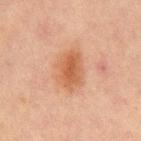Clinical impression:
Recorded during total-body skin imaging; not selected for excision or biopsy.
Clinical summary:
A close-up tile cropped from a whole-body skin photograph, about 15 mm across. Automated tile analysis of the lesion measured a lesion area of about 11 mm² and an eccentricity of roughly 0.7. The analysis additionally found a mean CIELAB color near L≈47 a*≈21 b*≈31, about 8 CIELAB-L* units darker than the surrounding skin, and a normalized border contrast of about 7.5. And it measured a nevus-likeness score of about 85/100 and a lesion-detection confidence of about 100/100. The lesion is on the mid back. Measured at roughly 4.5 mm in maximum diameter. This is a cross-polarized tile. The patient is a male roughly 65 years of age.